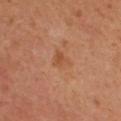notes: total-body-photography surveillance lesion; no biopsy
patient: female, in their 40s
image-analysis metrics: a within-lesion color-variation index near 2.5/10 and radial color variation of about 1; a classifier nevus-likeness of about 0/100 and a lesion-detection confidence of about 100/100
diameter: ~3 mm (longest diameter)
lighting: cross-polarized
image source: ~15 mm crop, total-body skin-cancer survey
site: the upper back